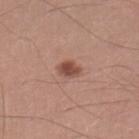On the leg.
Captured under white-light illumination.
An algorithmic analysis of the crop reported a border-irregularity index near 1.5/10, internal color variation of about 2.5 on a 0–10 scale, and a peripheral color-asymmetry measure near 1.
About 3 mm across.
A male subject aged 38–42.
A 15 mm crop from a total-body photograph taken for skin-cancer surveillance.